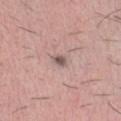Clinical impression: No biopsy was performed on this lesion — it was imaged during a full skin examination and was not determined to be concerning. Clinical summary: This is a white-light tile. Cropped from a whole-body photographic skin survey; the tile spans about 15 mm. The lesion-visualizer software estimated an average lesion color of about L≈54 a*≈16 b*≈20 (CIELAB), roughly 13 lightness units darker than nearby skin, and a lesion-to-skin contrast of about 8.5 (normalized; higher = more distinct). On the chest. The subject is a male approximately 30 years of age.